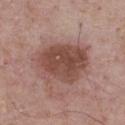Findings:
– workup — imaged on a skin check; not biopsied
– location — the chest
– subject — male, aged approximately 75
– imaging modality — 15 mm crop, total-body photography
– diameter — ~6.5 mm (longest diameter)
– image-analysis metrics — a lesion-to-skin contrast of about 8.5 (normalized; higher = more distinct); a border-irregularity rating of about 2.5/10, a color-variation rating of about 4.5/10, and a peripheral color-asymmetry measure near 1.5; a nevus-likeness score of about 75/100 and lesion-presence confidence of about 100/100